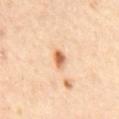{
  "biopsy_status": "not biopsied; imaged during a skin examination",
  "image": {
    "source": "total-body photography crop",
    "field_of_view_mm": 15
  },
  "site": "back",
  "patient": {
    "sex": "female",
    "age_approx": 60
  }
}